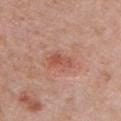Impression:
Recorded during total-body skin imaging; not selected for excision or biopsy.
Image and clinical context:
This is a white-light tile. A 15 mm close-up extracted from a 3D total-body photography capture. The total-body-photography lesion software estimated a footprint of about 4 mm². Located on the chest. Approximately 3 mm at its widest. A female patient aged approximately 50.